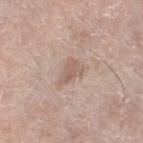Assessment:
The lesion was photographed on a routine skin check and not biopsied; there is no pathology result.
Background:
Automated tile analysis of the lesion measured a shape eccentricity near 0.75 and two-axis asymmetry of about 0.45. The lesion is on the left lower leg. A 15 mm close-up extracted from a 3D total-body photography capture. A female subject aged approximately 60. This is a white-light tile.